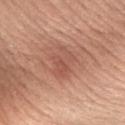Recorded during total-body skin imaging; not selected for excision or biopsy. The lesion-visualizer software estimated a lesion area of about 8.5 mm², a shape eccentricity near 0.65, and two-axis asymmetry of about 0.35. And it measured a lesion color around L≈54 a*≈25 b*≈29 in CIELAB, a lesion–skin lightness drop of about 7, and a normalized lesion–skin contrast near 5. It also reported a border-irregularity index near 4.5/10, internal color variation of about 4 on a 0–10 scale, and a peripheral color-asymmetry measure near 1.5. The analysis additionally found a nevus-likeness score of about 15/100 and a lesion-detection confidence of about 100/100. A 15 mm close-up extracted from a 3D total-body photography capture. A female patient, approximately 45 years of age. The tile uses white-light illumination. The lesion is on the arm. About 3.5 mm across.